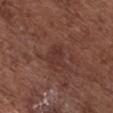Notes:
- follow-up · catalogued during a skin exam; not biopsied
- size · about 3 mm
- automated metrics · a mean CIELAB color near L≈34 a*≈21 b*≈23, about 5 CIELAB-L* units darker than the surrounding skin, and a lesion-to-skin contrast of about 5 (normalized; higher = more distinct); a border-irregularity rating of about 4.5/10, internal color variation of about 2 on a 0–10 scale, and radial color variation of about 0.5; a classifier nevus-likeness of about 0/100 and a lesion-detection confidence of about 100/100
- patient · female, approximately 80 years of age
- body site · the chest
- tile lighting · white-light illumination
- imaging modality · ~15 mm crop, total-body skin-cancer survey